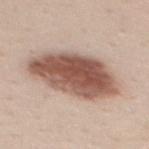Clinical summary: The lesion is located on the back. A roughly 15 mm field-of-view crop from a total-body skin photograph. The patient is a female aged around 25.